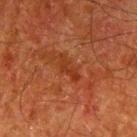An algorithmic analysis of the crop reported a border-irregularity rating of about 5/10 and a peripheral color-asymmetry measure near 0. The software also gave a nevus-likeness score of about 0/100 and a detector confidence of about 100 out of 100 that the crop contains a lesion.
From the left upper arm.
A 15 mm crop from a total-body photograph taken for skin-cancer surveillance.
A male subject, approximately 80 years of age.
Measured at roughly 3.5 mm in maximum diameter.